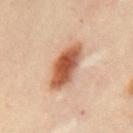The lesion was tiled from a total-body skin photograph and was not biopsied. The lesion is on the mid back. A male subject, aged approximately 50. This is a cross-polarized tile. A roughly 15 mm field-of-view crop from a total-body skin photograph. The recorded lesion diameter is about 5.5 mm.